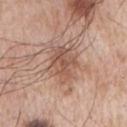Imaged during a routine full-body skin examination; the lesion was not biopsied and no histopathology is available.
The tile uses white-light illumination.
Cropped from a total-body skin-imaging series; the visible field is about 15 mm.
A male subject in their mid-60s.
The lesion is on the right upper arm.
Approximately 5 mm at its widest.
The lesion-visualizer software estimated a lesion area of about 10 mm², an eccentricity of roughly 0.8, and two-axis asymmetry of about 0.3. The analysis additionally found a mean CIELAB color near L≈54 a*≈20 b*≈28, about 10 CIELAB-L* units darker than the surrounding skin, and a normalized lesion–skin contrast near 6.5. It also reported a border-irregularity index near 3.5/10, a color-variation rating of about 3.5/10, and peripheral color asymmetry of about 1. The analysis additionally found lesion-presence confidence of about 100/100.